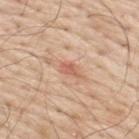Imaged during a routine full-body skin examination; the lesion was not biopsied and no histopathology is available. Approximately 2.5 mm at its widest. The tile uses white-light illumination. A male patient about 70 years old. The lesion is on the upper back. A 15 mm close-up extracted from a 3D total-body photography capture.